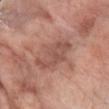subject = female, aged around 65 | image source = total-body-photography crop, ~15 mm field of view | lesion size = ~5 mm (longest diameter) | illumination = white-light illumination | anatomic site = the right forearm.